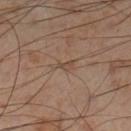Captured during whole-body skin photography for melanoma surveillance; the lesion was not biopsied. Approximately 2.5 mm at its widest. Imaged with cross-polarized lighting. A male subject approximately 55 years of age. A 15 mm crop from a total-body photograph taken for skin-cancer surveillance. The lesion is located on the left lower leg.